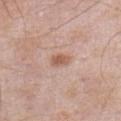Clinical impression:
Captured during whole-body skin photography for melanoma surveillance; the lesion was not biopsied.
Clinical summary:
From the abdomen. A lesion tile, about 15 mm wide, cut from a 3D total-body photograph. A male patient, roughly 55 years of age.